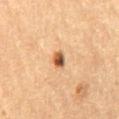Impression: Captured during whole-body skin photography for melanoma surveillance; the lesion was not biopsied. Image and clinical context: A female patient, aged 58 to 62. Cropped from a total-body skin-imaging series; the visible field is about 15 mm. From the lower back.